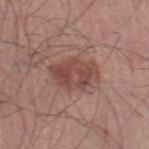Impression: No biopsy was performed on this lesion — it was imaged during a full skin examination and was not determined to be concerning. Acquisition and patient details: Captured under white-light illumination. A region of skin cropped from a whole-body photographic capture, roughly 15 mm wide. Automated tile analysis of the lesion measured an area of roughly 14 mm², a shape eccentricity near 0.7, and two-axis asymmetry of about 0.2. It also reported a mean CIELAB color near L≈47 a*≈21 b*≈24 and a normalized lesion–skin contrast near 7. The analysis additionally found internal color variation of about 4 on a 0–10 scale and radial color variation of about 1.5. The patient is a male aged 23 to 27. About 5 mm across. Located on the right lower leg.